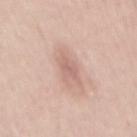workup = total-body-photography surveillance lesion; no biopsy
lighting = white-light
diameter = ≈4.5 mm
patient = male, about 45 years old
body site = the mid back
image source = ~15 mm crop, total-body skin-cancer survey